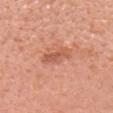Assessment:
Recorded during total-body skin imaging; not selected for excision or biopsy.
Acquisition and patient details:
A female patient in their mid-60s. Located on the head or neck. The tile uses white-light illumination. A lesion tile, about 15 mm wide, cut from a 3D total-body photograph.